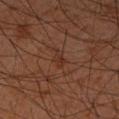No biopsy was performed on this lesion — it was imaged during a full skin examination and was not determined to be concerning. A region of skin cropped from a whole-body photographic capture, roughly 15 mm wide. This is a cross-polarized tile. A male patient, aged 58 to 62. From the left forearm.